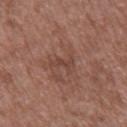Q: Was this lesion biopsied?
A: catalogued during a skin exam; not biopsied
Q: What are the patient's age and sex?
A: male, roughly 50 years of age
Q: Lesion location?
A: the back
Q: Lesion size?
A: ≈3 mm
Q: Illumination type?
A: white-light illumination
Q: What is the imaging modality?
A: total-body-photography crop, ~15 mm field of view